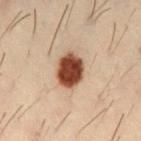Impression: The lesion was photographed on a routine skin check and not biopsied; there is no pathology result. Context: The lesion is on the right thigh. The patient is a male roughly 30 years of age. This image is a 15 mm lesion crop taken from a total-body photograph. The total-body-photography lesion software estimated a footprint of about 11 mm², an outline eccentricity of about 0.6 (0 = round, 1 = elongated), and a symmetry-axis asymmetry near 0.1. The tile uses cross-polarized illumination.